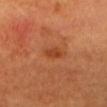Clinical impression: Captured during whole-body skin photography for melanoma surveillance; the lesion was not biopsied. Clinical summary: A roughly 15 mm field-of-view crop from a total-body skin photograph. A patient roughly 70 years of age. The total-body-photography lesion software estimated a footprint of about 3 mm², an eccentricity of roughly 0.8, and a symmetry-axis asymmetry near 0.3. On the head or neck.